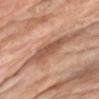biopsy status=no biopsy performed (imaged during a skin exam) | body site=the chest | automated lesion analysis=lesion-presence confidence of about 75/100 | patient=male, about 75 years old | size=about 5.5 mm | image source=~15 mm crop, total-body skin-cancer survey | lighting=white-light illumination.